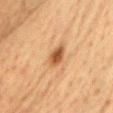Assessment: Imaged during a routine full-body skin examination; the lesion was not biopsied and no histopathology is available. Acquisition and patient details: The subject is a female aged around 55. The lesion-visualizer software estimated a border-irregularity index near 2.5/10, internal color variation of about 3.5 on a 0–10 scale, and radial color variation of about 1. It also reported a classifier nevus-likeness of about 95/100. A 15 mm close-up tile from a total-body photography series done for melanoma screening. The lesion's longest dimension is about 3 mm. Located on the head or neck. This is a cross-polarized tile.